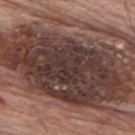workup=imaged on a skin check; not biopsied | site=the upper back | subject=male, aged approximately 80 | tile lighting=white-light | acquisition=total-body-photography crop, ~15 mm field of view | automated metrics=an eccentricity of roughly 0.9 and two-axis asymmetry of about 0.2; a mean CIELAB color near L≈39 a*≈18 b*≈21, about 17 CIELAB-L* units darker than the surrounding skin, and a normalized border contrast of about 13.